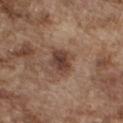A lesion tile, about 15 mm wide, cut from a 3D total-body photograph. The total-body-photography lesion software estimated an automated nevus-likeness rating near 75 out of 100 and a lesion-detection confidence of about 100/100. The lesion is located on the chest. Imaged with white-light lighting. A male patient, aged 73–77.